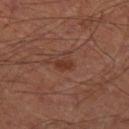Assessment: This lesion was catalogued during total-body skin photography and was not selected for biopsy. Background: A male patient about 60 years old. Approximately 2 mm at its widest. A close-up tile cropped from a whole-body skin photograph, about 15 mm across. The lesion is located on the leg. The lesion-visualizer software estimated border irregularity of about 2.5 on a 0–10 scale, internal color variation of about 1 on a 0–10 scale, and radial color variation of about 0.5.